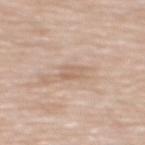  lighting: white-light
  image:
    source: total-body photography crop
    field_of_view_mm: 15
  site: mid back
  lesion_size:
    long_diameter_mm_approx: 2.5
  patient:
    sex: male
    age_approx: 70
  automated_metrics:
    cielab_L: 63
    cielab_a: 17
    cielab_b: 29
    vs_skin_darker_L: 7.0
    vs_skin_contrast_norm: 5.0
    nevus_likeness_0_100: 0
    lesion_detection_confidence_0_100: 100